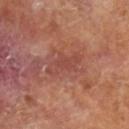| feature | finding |
|---|---|
| follow-up | total-body-photography surveillance lesion; no biopsy |
| subject | male, in their mid- to late 60s |
| lesion diameter | ≈4.5 mm |
| imaging modality | ~15 mm tile from a whole-body skin photo |
| image-analysis metrics | a footprint of about 7 mm², a shape eccentricity near 0.9, and two-axis asymmetry of about 0.4 |